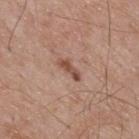The lesion was tiled from a total-body skin photograph and was not biopsied. Measured at roughly 3 mm in maximum diameter. The patient is a male roughly 70 years of age. From the upper back. A close-up tile cropped from a whole-body skin photograph, about 15 mm across. The tile uses white-light illumination.